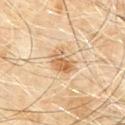A male patient, in their 60s.
This image is a 15 mm lesion crop taken from a total-body photograph.
On the mid back.
Imaged with cross-polarized lighting.
Approximately 3 mm at its widest.